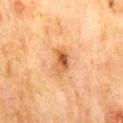The lesion was photographed on a routine skin check and not biopsied; there is no pathology result.
The lesion is on the mid back.
A male patient, roughly 70 years of age.
A close-up tile cropped from a whole-body skin photograph, about 15 mm across.
Imaged with cross-polarized lighting.
About 3 mm across.